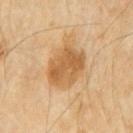A 15 mm close-up tile from a total-body photography series done for melanoma screening. The patient is a male aged around 60. The tile uses cross-polarized illumination. Longest diameter approximately 5.5 mm. The lesion is on the abdomen.